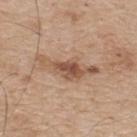The lesion was tiled from a total-body skin photograph and was not biopsied.
The tile uses white-light illumination.
A male subject in their mid-70s.
Located on the upper back.
A 15 mm close-up tile from a total-body photography series done for melanoma screening.
The recorded lesion diameter is about 7 mm.